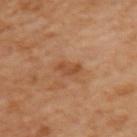biopsy status = catalogued during a skin exam; not biopsied
patient = female, aged approximately 55
lesion size = ≈2.5 mm
image-analysis metrics = a footprint of about 3.5 mm², a shape eccentricity near 0.8, and two-axis asymmetry of about 0.35; a mean CIELAB color near L≈50 a*≈23 b*≈37; a border-irregularity rating of about 3.5/10, a color-variation rating of about 2.5/10, and radial color variation of about 1
image source = ~15 mm tile from a whole-body skin photo
body site = the left upper arm